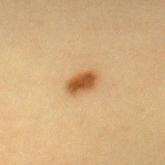biopsy status — imaged on a skin check; not biopsied
anatomic site — the upper back
TBP lesion metrics — an area of roughly 5 mm², an outline eccentricity of about 0.8 (0 = round, 1 = elongated), and a shape-asymmetry score of about 0.2 (0 = symmetric)
tile lighting — cross-polarized
acquisition — total-body-photography crop, ~15 mm field of view
patient — female, aged around 30